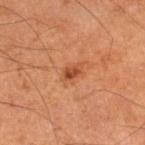workup = imaged on a skin check; not biopsied | image = total-body-photography crop, ~15 mm field of view | automated metrics = an area of roughly 3 mm², an eccentricity of roughly 0.8, and two-axis asymmetry of about 0.3; an average lesion color of about L≈49 a*≈29 b*≈38 (CIELAB), a lesion–skin lightness drop of about 11, and a normalized border contrast of about 7.5; a border-irregularity rating of about 2.5/10, a color-variation rating of about 2/10, and peripheral color asymmetry of about 0.5 | patient = male, roughly 60 years of age | lesion size = ≈2.5 mm | site = the right lower leg.